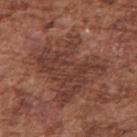Q: Was this lesion biopsied?
A: no biopsy performed (imaged during a skin exam)
Q: What is the imaging modality?
A: total-body-photography crop, ~15 mm field of view
Q: What is the lesion's diameter?
A: ≈7.5 mm
Q: Lesion location?
A: the right upper arm
Q: Patient demographics?
A: male, roughly 75 years of age
Q: What did automated image analysis measure?
A: a footprint of about 33 mm², a shape eccentricity near 0.55, and two-axis asymmetry of about 0.35; a lesion color around L≈39 a*≈22 b*≈25 in CIELAB and roughly 7 lightness units darker than nearby skin; a border-irregularity rating of about 7.5/10, a within-lesion color-variation index near 4/10, and a peripheral color-asymmetry measure near 1.5; a nevus-likeness score of about 0/100 and a detector confidence of about 85 out of 100 that the crop contains a lesion
Q: How was the tile lit?
A: white-light illumination